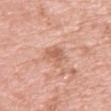| field | value |
|---|---|
| imaging modality | 15 mm crop, total-body photography |
| anatomic site | the front of the torso |
| patient | female, aged approximately 70 |